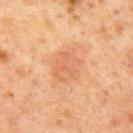Captured during whole-body skin photography for melanoma surveillance; the lesion was not biopsied. On the mid back. An algorithmic analysis of the crop reported an area of roughly 11 mm² and a shape eccentricity near 0.5. The software also gave roughly 6 lightness units darker than nearby skin. It also reported a classifier nevus-likeness of about 25/100 and a detector confidence of about 100 out of 100 that the crop contains a lesion. This is a cross-polarized tile. The patient is a male aged 63 to 67. Cropped from a whole-body photographic skin survey; the tile spans about 15 mm. Approximately 4.5 mm at its widest.